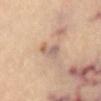The lesion was photographed on a routine skin check and not biopsied; there is no pathology result. The recorded lesion diameter is about 3 mm. Located on the chest. A roughly 15 mm field-of-view crop from a total-body skin photograph.